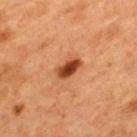<tbp_lesion>
<biopsy_status>not biopsied; imaged during a skin examination</biopsy_status>
<image>
  <source>total-body photography crop</source>
  <field_of_view_mm>15</field_of_view_mm>
</image>
<lesion_size>
  <long_diameter_mm_approx>3.0</long_diameter_mm_approx>
</lesion_size>
<site>mid back</site>
<automated_metrics>
  <cielab_L>38</cielab_L>
  <cielab_a>27</cielab_a>
  <cielab_b>35</cielab_b>
  <vs_skin_darker_L>16.0</vs_skin_darker_L>
  <vs_skin_contrast_norm>12.0</vs_skin_contrast_norm>
  <nevus_likeness_0_100>95</nevus_likeness_0_100>
</automated_metrics>
<patient>
  <sex>female</sex>
  <age_approx>40</age_approx>
</patient>
</tbp_lesion>